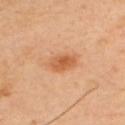<record>
<biopsy_status>not biopsied; imaged during a skin examination</biopsy_status>
<lesion_size>
  <long_diameter_mm_approx>3.5</long_diameter_mm_approx>
</lesion_size>
<patient>
  <sex>male</sex>
  <age_approx>50</age_approx>
</patient>
<image>
  <source>total-body photography crop</source>
  <field_of_view_mm>15</field_of_view_mm>
</image>
<site>upper back</site>
</record>